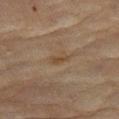Q: Is there a histopathology result?
A: catalogued during a skin exam; not biopsied
Q: What kind of image is this?
A: total-body-photography crop, ~15 mm field of view
Q: Lesion size?
A: ≈2.5 mm
Q: What did automated image analysis measure?
A: a footprint of about 2.5 mm² and a shape-asymmetry score of about 0.35 (0 = symmetric); about 5 CIELAB-L* units darker than the surrounding skin and a normalized border contrast of about 5.5; a border-irregularity rating of about 3.5/10, a color-variation rating of about 0/10, and a peripheral color-asymmetry measure near 0
Q: Patient demographics?
A: female, aged 78–82
Q: Lesion location?
A: the left thigh